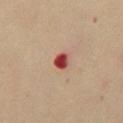notes: no biopsy performed (imaged during a skin exam)
illumination: cross-polarized
site: the chest
imaging modality: total-body-photography crop, ~15 mm field of view
automated metrics: a footprint of about 3.5 mm², an eccentricity of roughly 0.75, and two-axis asymmetry of about 0.25; about 18 CIELAB-L* units darker than the surrounding skin and a normalized border contrast of about 13; a border-irregularity rating of about 2/10, a color-variation rating of about 2.5/10, and radial color variation of about 0.5; a lesion-detection confidence of about 100/100
lesion size: ≈2.5 mm
patient: male, approximately 50 years of age